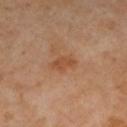{"biopsy_status": "not biopsied; imaged during a skin examination", "automated_metrics": {"area_mm2_approx": 4.5, "eccentricity": 0.8, "shape_asymmetry": 0.3, "cielab_L": 51, "cielab_a": 24, "cielab_b": 36, "vs_skin_darker_L": 8.0, "vs_skin_contrast_norm": 6.5, "border_irregularity_0_10": 3.0, "color_variation_0_10": 1.5, "peripheral_color_asymmetry": 0.5, "nevus_likeness_0_100": 0, "lesion_detection_confidence_0_100": 100}, "patient": {"sex": "female", "age_approx": 50}, "site": "right lower leg", "image": {"source": "total-body photography crop", "field_of_view_mm": 15}, "lighting": "cross-polarized", "lesion_size": {"long_diameter_mm_approx": 3.0}}